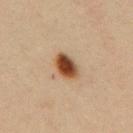Assessment:
Recorded during total-body skin imaging; not selected for excision or biopsy.
Context:
Automated tile analysis of the lesion measured an average lesion color of about L≈41 a*≈19 b*≈31 (CIELAB), a lesion–skin lightness drop of about 17, and a lesion-to-skin contrast of about 13 (normalized; higher = more distinct). And it measured a border-irregularity rating of about 2/10. It also reported an automated nevus-likeness rating near 100 out of 100 and a detector confidence of about 100 out of 100 that the crop contains a lesion. The tile uses cross-polarized illumination. This image is a 15 mm lesion crop taken from a total-body photograph. From the upper back. Approximately 3.5 mm at its widest. A female subject, aged around 45.